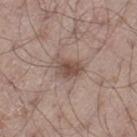notes = catalogued during a skin exam; not biopsied
body site = the leg
patient = male, aged around 55
acquisition = ~15 mm tile from a whole-body skin photo
image-analysis metrics = a lesion area of about 6.5 mm²; a border-irregularity index near 4/10 and a peripheral color-asymmetry measure near 1
size = ≈3.5 mm
tile lighting = white-light